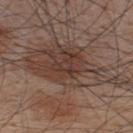Clinical summary: A region of skin cropped from a whole-body photographic capture, roughly 15 mm wide. A male subject, aged 33–37. The recorded lesion diameter is about 13 mm. Automated image analysis of the tile measured an average lesion color of about L≈40 a*≈16 b*≈23 (CIELAB), about 9 CIELAB-L* units darker than the surrounding skin, and a normalized lesion–skin contrast near 7.5. Captured under white-light illumination. On the upper back.